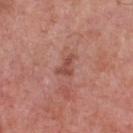Recorded during total-body skin imaging; not selected for excision or biopsy. A 15 mm close-up extracted from a 3D total-body photography capture. A male patient, aged 68–72. The recorded lesion diameter is about 3 mm. The tile uses white-light illumination. On the front of the torso. Automated image analysis of the tile measured a lesion color around L≈49 a*≈26 b*≈27 in CIELAB, a lesion–skin lightness drop of about 9, and a normalized border contrast of about 7. It also reported a border-irregularity rating of about 5/10, a within-lesion color-variation index near 1.5/10, and peripheral color asymmetry of about 0.5.